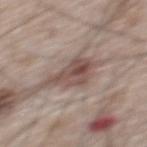The lesion was tiled from a total-body skin photograph and was not biopsied. Longest diameter approximately 4.5 mm. Imaged with white-light lighting. A 15 mm close-up extracted from a 3D total-body photography capture. A male patient, roughly 65 years of age. The lesion is on the upper back.